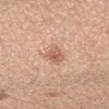<lesion>
<biopsy_status>not biopsied; imaged during a skin examination</biopsy_status>
<site>right forearm</site>
<automated_metrics>
  <nevus_likeness_0_100>75</nevus_likeness_0_100>
  <lesion_detection_confidence_0_100>100</lesion_detection_confidence_0_100>
</automated_metrics>
<image>
  <source>total-body photography crop</source>
  <field_of_view_mm>15</field_of_view_mm>
</image>
<lesion_size>
  <long_diameter_mm_approx>3.5</long_diameter_mm_approx>
</lesion_size>
<patient>
  <sex>female</sex>
  <age_approx>40</age_approx>
</patient>
</lesion>